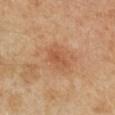Assessment: Recorded during total-body skin imaging; not selected for excision or biopsy. Acquisition and patient details: Automated tile analysis of the lesion measured an outline eccentricity of about 0.75 (0 = round, 1 = elongated) and a shape-asymmetry score of about 0.3 (0 = symmetric). The software also gave roughly 7 lightness units darker than nearby skin and a normalized lesion–skin contrast near 5.5. From the left upper arm. A 15 mm close-up tile from a total-body photography series done for melanoma screening. The tile uses cross-polarized illumination. A female subject about 40 years old. The lesion's longest dimension is about 2.5 mm.